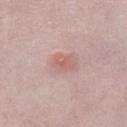follow-up: no biopsy performed (imaged during a skin exam); acquisition: 15 mm crop, total-body photography; subject: female, roughly 45 years of age; body site: the left lower leg; illumination: white-light.